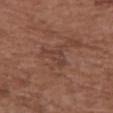workup = no biopsy performed (imaged during a skin exam)
size = ~3.5 mm (longest diameter)
location = the chest
lighting = white-light illumination
imaging modality = total-body-photography crop, ~15 mm field of view
TBP lesion metrics = an eccentricity of roughly 0.85 and a symmetry-axis asymmetry near 0.55; border irregularity of about 6 on a 0–10 scale, a within-lesion color-variation index near 1.5/10, and a peripheral color-asymmetry measure near 0.5
patient = female, approximately 75 years of age